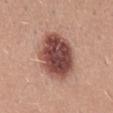| feature | finding |
|---|---|
| follow-up | imaged on a skin check; not biopsied |
| body site | the mid back |
| illumination | white-light illumination |
| subject | male, aged 23–27 |
| image | ~15 mm crop, total-body skin-cancer survey |
| diameter | about 6.5 mm |